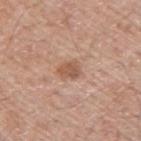Part of a total-body skin-imaging series; this lesion was reviewed on a skin check and was not flagged for biopsy. A male patient aged 53–57. The lesion is on the upper back. A close-up tile cropped from a whole-body skin photograph, about 15 mm across.